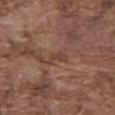Assessment: Recorded during total-body skin imaging; not selected for excision or biopsy. Context: A male subject approximately 75 years of age. Located on the abdomen. Measured at roughly 3 mm in maximum diameter. The lesion-visualizer software estimated a within-lesion color-variation index near 0.5/10 and peripheral color asymmetry of about 0. It also reported an automated nevus-likeness rating near 0 out of 100 and a detector confidence of about 65 out of 100 that the crop contains a lesion. This image is a 15 mm lesion crop taken from a total-body photograph.